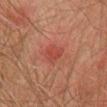The tile uses cross-polarized illumination. From the chest. A 15 mm close-up extracted from a 3D total-body photography capture. A male subject in their mid-70s.